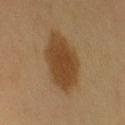Notes:
* notes · total-body-photography surveillance lesion; no biopsy
* automated lesion analysis · a lesion color around L≈43 a*≈17 b*≈34 in CIELAB and roughly 11 lightness units darker than nearby skin; a border-irregularity rating of about 1.5/10, internal color variation of about 2.5 on a 0–10 scale, and peripheral color asymmetry of about 0.5
* location · the left upper arm
* image · 15 mm crop, total-body photography
* subject · male, aged 58–62
* tile lighting · cross-polarized illumination